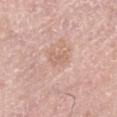Recorded during total-body skin imaging; not selected for excision or biopsy. Cropped from a whole-body photographic skin survey; the tile spans about 15 mm. This is a white-light tile. Automated image analysis of the tile measured a shape eccentricity near 0.9 and a symmetry-axis asymmetry near 0.35. It also reported a mean CIELAB color near L≈64 a*≈20 b*≈29, a lesion–skin lightness drop of about 7, and a lesion-to-skin contrast of about 5 (normalized; higher = more distinct). The analysis additionally found an automated nevus-likeness rating near 0 out of 100 and lesion-presence confidence of about 100/100. The patient is a male in their 60s. The lesion's longest dimension is about 3 mm. Located on the right lower leg.